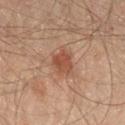Part of a total-body skin-imaging series; this lesion was reviewed on a skin check and was not flagged for biopsy.
A close-up tile cropped from a whole-body skin photograph, about 15 mm across.
Measured at roughly 3 mm in maximum diameter.
From the leg.
The subject is a male aged 63 to 67.